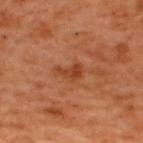Q: Was this lesion biopsied?
A: catalogued during a skin exam; not biopsied
Q: What is the lesion's diameter?
A: ~3 mm (longest diameter)
Q: What kind of image is this?
A: ~15 mm crop, total-body skin-cancer survey
Q: What lighting was used for the tile?
A: cross-polarized
Q: Automated lesion metrics?
A: a lesion area of about 3.5 mm², an outline eccentricity of about 0.8 (0 = round, 1 = elongated), and two-axis asymmetry of about 0.55; a lesion color around L≈43 a*≈29 b*≈38 in CIELAB and a normalized lesion–skin contrast near 7.5; a border-irregularity rating of about 5/10, a color-variation rating of about 2/10, and radial color variation of about 0.5; a nevus-likeness score of about 0/100 and a lesion-detection confidence of about 100/100
Q: Lesion location?
A: the upper back
Q: Who is the patient?
A: male, aged approximately 50